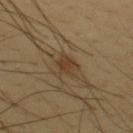Assessment:
This lesion was catalogued during total-body skin photography and was not selected for biopsy.
Clinical summary:
The recorded lesion diameter is about 3 mm. A region of skin cropped from a whole-body photographic capture, roughly 15 mm wide. This is a cross-polarized tile. On the upper back. An algorithmic analysis of the crop reported an area of roughly 6 mm², a shape eccentricity near 0.6, and a symmetry-axis asymmetry near 0.35. A male subject, about 45 years old.